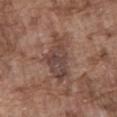workup = total-body-photography surveillance lesion; no biopsy.